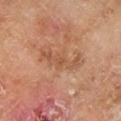Background: Captured under cross-polarized illumination. The lesion's longest dimension is about 5.5 mm. A lesion tile, about 15 mm wide, cut from a 3D total-body photograph. Automated tile analysis of the lesion measured a lesion area of about 7 mm², an outline eccentricity of about 0.95 (0 = round, 1 = elongated), and a shape-asymmetry score of about 0.45 (0 = symmetric). And it measured an average lesion color of about L≈54 a*≈23 b*≈34 (CIELAB), about 7 CIELAB-L* units darker than the surrounding skin, and a normalized lesion–skin contrast near 5. It also reported internal color variation of about 1.5 on a 0–10 scale and radial color variation of about 0.5. The subject is a male about 65 years old. The lesion is located on the right lower leg.